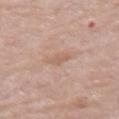Q: What is the imaging modality?
A: total-body-photography crop, ~15 mm field of view
Q: What are the patient's age and sex?
A: female, in their mid-60s
Q: How large is the lesion?
A: ~2.5 mm (longest diameter)
Q: Lesion location?
A: the arm
Q: Illumination type?
A: white-light